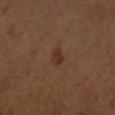Impression: Recorded during total-body skin imaging; not selected for excision or biopsy. Context: Cropped from a whole-body photographic skin survey; the tile spans about 15 mm. The lesion's longest dimension is about 2.5 mm. A female subject, about 55 years old. This is a cross-polarized tile. On the right forearm.